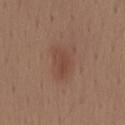Q: Was this lesion biopsied?
A: no biopsy performed (imaged during a skin exam)
Q: What did automated image analysis measure?
A: a footprint of about 6 mm², an eccentricity of roughly 0.85, and a shape-asymmetry score of about 0.3 (0 = symmetric); a lesion color around L≈44 a*≈20 b*≈27 in CIELAB and about 7 CIELAB-L* units darker than the surrounding skin; border irregularity of about 3 on a 0–10 scale, internal color variation of about 2.5 on a 0–10 scale, and a peripheral color-asymmetry measure near 1; a classifier nevus-likeness of about 85/100 and a lesion-detection confidence of about 100/100
Q: Who is the patient?
A: male, roughly 40 years of age
Q: How large is the lesion?
A: ~4 mm (longest diameter)
Q: What is the imaging modality?
A: total-body-photography crop, ~15 mm field of view
Q: Where on the body is the lesion?
A: the mid back